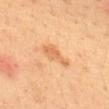Q: Patient demographics?
A: male, aged around 35
Q: Where on the body is the lesion?
A: the upper back
Q: Illumination type?
A: cross-polarized
Q: What is the imaging modality?
A: total-body-photography crop, ~15 mm field of view
Q: How large is the lesion?
A: about 3.5 mm